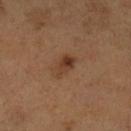biopsy status = catalogued during a skin exam; not biopsied
illumination = cross-polarized illumination
image = 15 mm crop, total-body photography
patient = male, roughly 55 years of age
lesion size = ≈3.5 mm
location = the leg